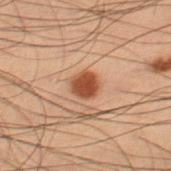Imaged during a routine full-body skin examination; the lesion was not biopsied and no histopathology is available. A close-up tile cropped from a whole-body skin photograph, about 15 mm across. The lesion's longest dimension is about 2.5 mm. The lesion is located on the right thigh. The subject is a male aged approximately 55.